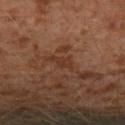Clinical impression: The lesion was tiled from a total-body skin photograph and was not biopsied. Image and clinical context: A male subject aged approximately 30. The lesion is on the left lower leg. A 15 mm close-up tile from a total-body photography series done for melanoma screening. The tile uses cross-polarized illumination.